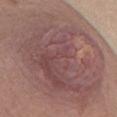Assessment: Imaged during a routine full-body skin examination; the lesion was not biopsied and no histopathology is available. Background: A female patient aged around 20. Cropped from a total-body skin-imaging series; the visible field is about 15 mm. Longest diameter approximately 14.5 mm. The total-body-photography lesion software estimated a footprint of about 110 mm², an outline eccentricity of about 0.7 (0 = round, 1 = elongated), and a symmetry-axis asymmetry near 0.1. The software also gave about 9 CIELAB-L* units darker than the surrounding skin and a lesion-to-skin contrast of about 7 (normalized; higher = more distinct). The software also gave a border-irregularity rating of about 1.5/10, a color-variation rating of about 6.5/10, and a peripheral color-asymmetry measure near 2. The analysis additionally found an automated nevus-likeness rating near 0 out of 100. Captured under white-light illumination. On the chest.